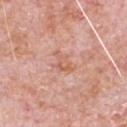Impression:
The lesion was tiled from a total-body skin photograph and was not biopsied.
Image and clinical context:
Cropped from a total-body skin-imaging series; the visible field is about 15 mm. A male subject approximately 80 years of age. On the chest.